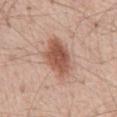The lesion was photographed on a routine skin check and not biopsied; there is no pathology result. Longest diameter approximately 5.5 mm. A male subject aged 53–57. This image is a 15 mm lesion crop taken from a total-body photograph. This is a white-light tile. The lesion is on the abdomen.